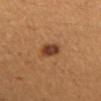This lesion was catalogued during total-body skin photography and was not selected for biopsy.
The lesion is on the left forearm.
A close-up tile cropped from a whole-body skin photograph, about 15 mm across.
The total-body-photography lesion software estimated an area of roughly 5 mm², an outline eccentricity of about 0.45 (0 = round, 1 = elongated), and two-axis asymmetry of about 0.15. The analysis additionally found a mean CIELAB color near L≈40 a*≈23 b*≈33.
Approximately 2.5 mm at its widest.
A female patient, in their mid- to late 20s.